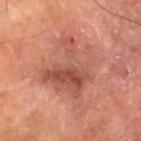lesion_size:
  long_diameter_mm_approx: 8.0
site: right thigh
patient:
  sex: male
  age_approx: 80
lighting: cross-polarized
image:
  source: total-body photography crop
  field_of_view_mm: 15